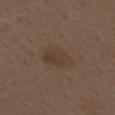Findings:
– notes: imaged on a skin check; not biopsied
– TBP lesion metrics: a footprint of about 8 mm²; border irregularity of about 2 on a 0–10 scale, a within-lesion color-variation index near 2.5/10, and peripheral color asymmetry of about 1
– body site: the mid back
– lesion diameter: ≈3.5 mm
– image: ~15 mm crop, total-body skin-cancer survey
– lighting: white-light
– subject: male, aged 68 to 72